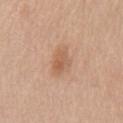Recorded during total-body skin imaging; not selected for excision or biopsy. Automated tile analysis of the lesion measured a lesion–skin lightness drop of about 8. And it measured border irregularity of about 3 on a 0–10 scale, internal color variation of about 3 on a 0–10 scale, and peripheral color asymmetry of about 1. The software also gave a lesion-detection confidence of about 100/100. Cropped from a total-body skin-imaging series; the visible field is about 15 mm. About 3 mm across. A female patient, aged approximately 45. On the front of the torso.